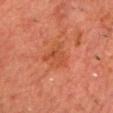Captured during whole-body skin photography for melanoma surveillance; the lesion was not biopsied.